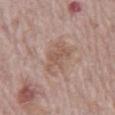The lesion was photographed on a routine skin check and not biopsied; there is no pathology result.